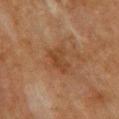biopsy_status: not biopsied; imaged during a skin examination
image:
  source: total-body photography crop
  field_of_view_mm: 15
site: upper back
patient:
  sex: female
  age_approx: 70
lighting: cross-polarized
lesion_size:
  long_diameter_mm_approx: 3.5
automated_metrics:
  area_mm2_approx: 6.0
  shape_asymmetry: 0.3
  vs_skin_darker_L: 6.0
  vs_skin_contrast_norm: 6.0